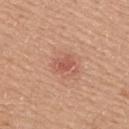Q: Was a biopsy performed?
A: imaged on a skin check; not biopsied
Q: What kind of image is this?
A: total-body-photography crop, ~15 mm field of view
Q: How large is the lesion?
A: ~2.5 mm (longest diameter)
Q: What lighting was used for the tile?
A: white-light illumination
Q: Lesion location?
A: the right upper arm
Q: Patient demographics?
A: male, roughly 20 years of age
Q: What did automated image analysis measure?
A: border irregularity of about 2 on a 0–10 scale, internal color variation of about 2.5 on a 0–10 scale, and radial color variation of about 1